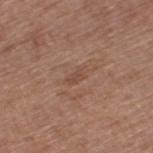{"biopsy_status": "not biopsied; imaged during a skin examination", "patient": {"sex": "female", "age_approx": 75}, "site": "leg", "image": {"source": "total-body photography crop", "field_of_view_mm": 15}, "lighting": "white-light", "lesion_size": {"long_diameter_mm_approx": 2.5}, "automated_metrics": {"eccentricity": 0.85, "shape_asymmetry": 0.3, "vs_skin_darker_L": 6.0, "vs_skin_contrast_norm": 5.0, "lesion_detection_confidence_0_100": 100}}